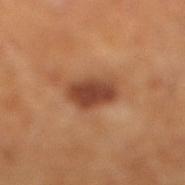The lesion was tiled from a total-body skin photograph and was not biopsied. This image is a 15 mm lesion crop taken from a total-body photograph. A male patient aged approximately 60. Located on the leg.